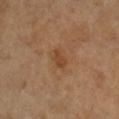Imaged during a routine full-body skin examination; the lesion was not biopsied and no histopathology is available. A female patient roughly 55 years of age. The lesion is on the right lower leg. The total-body-photography lesion software estimated a shape eccentricity near 0.8 and a symmetry-axis asymmetry near 0.3. The software also gave a border-irregularity index near 2.5/10, a within-lesion color-variation index near 1.5/10, and peripheral color asymmetry of about 0.5. A close-up tile cropped from a whole-body skin photograph, about 15 mm across. About 2.5 mm across.